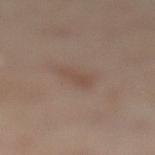About 3.5 mm across.
From the left lower leg.
The subject is a female about 55 years old.
An algorithmic analysis of the crop reported a footprint of about 4.5 mm² and two-axis asymmetry of about 0.35.
This image is a 15 mm lesion crop taken from a total-body photograph.